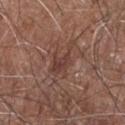No biopsy was performed on this lesion — it was imaged during a full skin examination and was not determined to be concerning.